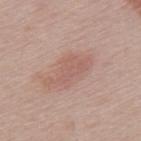This lesion was catalogued during total-body skin photography and was not selected for biopsy.